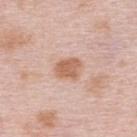| key | value |
|---|---|
| follow-up | imaged on a skin check; not biopsied |
| anatomic site | the upper back |
| patient | female, aged 63–67 |
| lesion diameter | ~3 mm (longest diameter) |
| tile lighting | white-light |
| acquisition | total-body-photography crop, ~15 mm field of view |